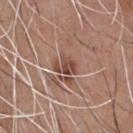The lesion was photographed on a routine skin check and not biopsied; there is no pathology result. From the chest. Longest diameter approximately 2.5 mm. A male patient aged 53 to 57. A 15 mm crop from a total-body photograph taken for skin-cancer surveillance.